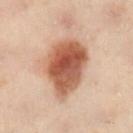Q: What are the patient's age and sex?
A: female, about 60 years old
Q: How was this image acquired?
A: total-body-photography crop, ~15 mm field of view
Q: How was the tile lit?
A: cross-polarized illumination
Q: What is the lesion's diameter?
A: ≈6.5 mm
Q: What is the anatomic site?
A: the left leg